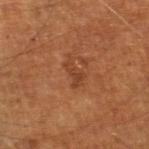Impression: The lesion was tiled from a total-body skin photograph and was not biopsied. Acquisition and patient details: A roughly 15 mm field-of-view crop from a total-body skin photograph. This is a cross-polarized tile. Measured at roughly 3 mm in maximum diameter. The lesion is located on the left leg. A male patient in their mid-60s.